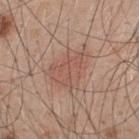The lesion was photographed on a routine skin check and not biopsied; there is no pathology result.
A region of skin cropped from a whole-body photographic capture, roughly 15 mm wide.
The lesion is located on the upper back.
A male subject about 50 years old.
The tile uses white-light illumination.